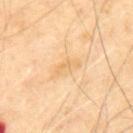Assessment:
Captured during whole-body skin photography for melanoma surveillance; the lesion was not biopsied.
Image and clinical context:
A 15 mm crop from a total-body photograph taken for skin-cancer surveillance. A male patient, approximately 70 years of age. The lesion is on the mid back. The lesion-visualizer software estimated a border-irregularity index near 7.5/10 and peripheral color asymmetry of about 0. It also reported a nevus-likeness score of about 0/100. About 3 mm across. Imaged with cross-polarized lighting.